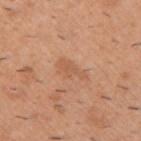* workup — no biopsy performed (imaged during a skin exam)
* body site — the right upper arm
* subject — male, aged 38 to 42
* size — ≈4 mm
* image — ~15 mm crop, total-body skin-cancer survey
* illumination — white-light